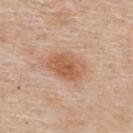Assessment:
The lesion was photographed on a routine skin check and not biopsied; there is no pathology result.
Clinical summary:
On the back. A male patient, aged approximately 55. Cropped from a total-body skin-imaging series; the visible field is about 15 mm.